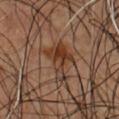biopsy status: catalogued during a skin exam; not biopsied | tile lighting: cross-polarized illumination | patient: male, in their mid- to late 40s | acquisition: 15 mm crop, total-body photography | body site: the chest | lesion diameter: ~4.5 mm (longest diameter).